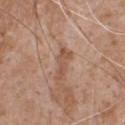Image and clinical context: The lesion's longest dimension is about 4 mm. A male subject, aged 63–67. A roughly 15 mm field-of-view crop from a total-body skin photograph. Imaged with white-light lighting. On the chest.